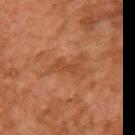The lesion was tiled from a total-body skin photograph and was not biopsied. The recorded lesion diameter is about 3 mm. The lesion is located on the right upper arm. A female patient, aged 58–62. Captured under cross-polarized illumination. A roughly 15 mm field-of-view crop from a total-body skin photograph.